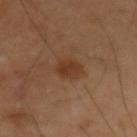Imaged during a routine full-body skin examination; the lesion was not biopsied and no histopathology is available. Approximately 2.5 mm at its widest. The patient is a male in their 50s. A roughly 15 mm field-of-view crop from a total-body skin photograph. On the left upper arm.